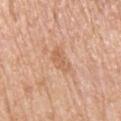{
  "site": "right upper arm",
  "lesion_size": {
    "long_diameter_mm_approx": 3.5
  },
  "image": {
    "source": "total-body photography crop",
    "field_of_view_mm": 15
  },
  "lighting": "white-light",
  "patient": {
    "sex": "male",
    "age_approx": 70
  }
}